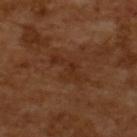No biopsy was performed on this lesion — it was imaged during a full skin examination and was not determined to be concerning.
Automated image analysis of the tile measured a shape eccentricity near 0.85 and a symmetry-axis asymmetry near 0.4. And it measured border irregularity of about 6 on a 0–10 scale and radial color variation of about 0.5. The analysis additionally found an automated nevus-likeness rating near 0 out of 100 and a lesion-detection confidence of about 95/100.
The patient is a male in their mid-60s.
This is a cross-polarized tile.
A 15 mm close-up tile from a total-body photography series done for melanoma screening.
The lesion's longest dimension is about 5 mm.